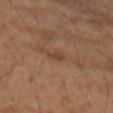Case summary:
* image-analysis metrics · a lesion area of about 2.5 mm² and a symmetry-axis asymmetry near 0.4; an average lesion color of about L≈43 a*≈20 b*≈31 (CIELAB), about 7 CIELAB-L* units darker than the surrounding skin, and a normalized border contrast of about 6
* body site · the right upper arm
* image · ~15 mm tile from a whole-body skin photo
* lighting · cross-polarized
* diameter · ~2.5 mm (longest diameter)
* patient · female, in their 60s